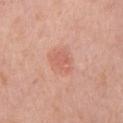The lesion was photographed on a routine skin check and not biopsied; there is no pathology result. Approximately 3 mm at its widest. From the arm. A 15 mm close-up extracted from a 3D total-body photography capture. The tile uses white-light illumination. An algorithmic analysis of the crop reported a border-irregularity rating of about 2.5/10 and internal color variation of about 2 on a 0–10 scale. It also reported a classifier nevus-likeness of about 35/100. A female subject roughly 70 years of age.